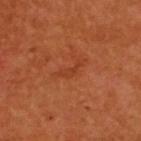Impression: The lesion was photographed on a routine skin check and not biopsied; there is no pathology result. Background: On the upper back. A female subject, aged 53–57. Cropped from a whole-body photographic skin survey; the tile spans about 15 mm.